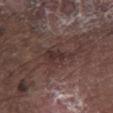notes = total-body-photography surveillance lesion; no biopsy | lighting = white-light illumination | diameter = ~4.5 mm (longest diameter) | subject = male, about 75 years old | location = the left lower leg | image = ~15 mm tile from a whole-body skin photo.